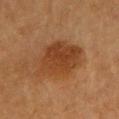Imaged during a routine full-body skin examination; the lesion was not biopsied and no histopathology is available. A region of skin cropped from a whole-body photographic capture, roughly 15 mm wide. Longest diameter approximately 6.5 mm. From the upper back. Automated image analysis of the tile measured a lesion area of about 21 mm², an outline eccentricity of about 0.65 (0 = round, 1 = elongated), and two-axis asymmetry of about 0.15. The software also gave border irregularity of about 2.5 on a 0–10 scale, internal color variation of about 3.5 on a 0–10 scale, and peripheral color asymmetry of about 1.5. The analysis additionally found an automated nevus-likeness rating near 85 out of 100 and a detector confidence of about 100 out of 100 that the crop contains a lesion. A female patient, in their 60s. Imaged with cross-polarized lighting.